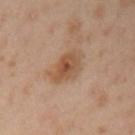  biopsy_status: not biopsied; imaged during a skin examination
  lesion_size:
    long_diameter_mm_approx: 4.5
  image:
    source: total-body photography crop
    field_of_view_mm: 15
  lighting: cross-polarized
  site: arm
  patient:
    sex: female
    age_approx: 40